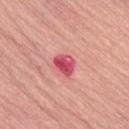- lighting — white-light illumination
- image source — ~15 mm crop, total-body skin-cancer survey
- patient — female, aged 63–67
- TBP lesion metrics — a lesion color around L≈54 a*≈43 b*≈22 in CIELAB; a border-irregularity index near 2.5/10 and a peripheral color-asymmetry measure near 1.5
- body site — the right thigh